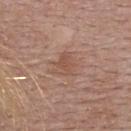biopsy status = total-body-photography surveillance lesion; no biopsy | TBP lesion metrics = a lesion area of about 5 mm²; a mean CIELAB color near L≈52 a*≈20 b*≈28 and a normalized border contrast of about 5; a nevus-likeness score of about 0/100 and a detector confidence of about 100 out of 100 that the crop contains a lesion | patient = female, roughly 50 years of age | anatomic site = the upper back | diameter = ~3 mm (longest diameter) | image source = total-body-photography crop, ~15 mm field of view.